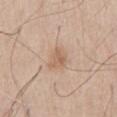Clinical impression:
The lesion was photographed on a routine skin check and not biopsied; there is no pathology result.
Context:
The subject is a male in their mid- to late 60s. Approximately 3 mm at its widest. The lesion is on the chest. Cropped from a whole-body photographic skin survey; the tile spans about 15 mm.